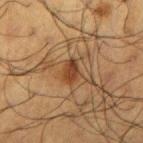Notes:
– follow-up: catalogued during a skin exam; not biopsied
– TBP lesion metrics: an area of roughly 5.5 mm², an outline eccentricity of about 0.55 (0 = round, 1 = elongated), and two-axis asymmetry of about 0.35; border irregularity of about 3 on a 0–10 scale and radial color variation of about 1.5; a detector confidence of about 100 out of 100 that the crop contains a lesion
– site: the right upper arm
– image source: 15 mm crop, total-body photography
– diameter: ~3 mm (longest diameter)
– subject: male, in their 60s